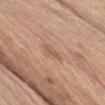notes: no biopsy performed (imaged during a skin exam) | diameter: ≈3.5 mm | image source: ~15 mm crop, total-body skin-cancer survey | patient: male, about 70 years old | site: the front of the torso | image-analysis metrics: a lesion–skin lightness drop of about 7; border irregularity of about 3.5 on a 0–10 scale, internal color variation of about 1 on a 0–10 scale, and a peripheral color-asymmetry measure near 0.5; a nevus-likeness score of about 0/100 and a detector confidence of about 100 out of 100 that the crop contains a lesion.